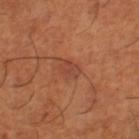Case summary:
– image-analysis metrics · a footprint of about 4.5 mm², an outline eccentricity of about 0.55 (0 = round, 1 = elongated), and a symmetry-axis asymmetry near 0.25; a lesion color around L≈44 a*≈26 b*≈32 in CIELAB, a lesion–skin lightness drop of about 6, and a normalized border contrast of about 5; border irregularity of about 2.5 on a 0–10 scale, internal color variation of about 2 on a 0–10 scale, and a peripheral color-asymmetry measure near 0.5
– imaging modality · ~15 mm tile from a whole-body skin photo
– subject · male, aged 63–67
– body site · the leg
– diameter · ≈2.5 mm